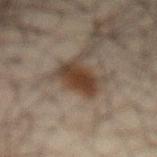Imaged during a routine full-body skin examination; the lesion was not biopsied and no histopathology is available. Captured under cross-polarized illumination. Approximately 3.5 mm at its widest. A male patient, about 65 years old. Located on the abdomen. A 15 mm close-up tile from a total-body photography series done for melanoma screening. The lesion-visualizer software estimated a lesion color around L≈29 a*≈13 b*≈22 in CIELAB and a lesion-to-skin contrast of about 11.5 (normalized; higher = more distinct). The software also gave internal color variation of about 2.5 on a 0–10 scale and a peripheral color-asymmetry measure near 0.5.